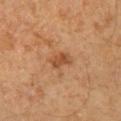Acquisition and patient details:
A male subject about 65 years old. This is a cross-polarized tile. Automated tile analysis of the lesion measured a lesion color around L≈44 a*≈22 b*≈33 in CIELAB, a lesion–skin lightness drop of about 9, and a normalized border contrast of about 7. It also reported a border-irregularity rating of about 2.5/10, a within-lesion color-variation index near 3/10, and radial color variation of about 1. It also reported a detector confidence of about 100 out of 100 that the crop contains a lesion. On the right lower leg. A region of skin cropped from a whole-body photographic capture, roughly 15 mm wide.